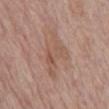Case summary:
* follow-up · total-body-photography surveillance lesion; no biopsy
* subject · male, aged approximately 70
* image-analysis metrics · an area of roughly 12 mm² and a shape-asymmetry score of about 0.45 (0 = symmetric); a border-irregularity rating of about 6/10, a within-lesion color-variation index near 3.5/10, and peripheral color asymmetry of about 1; a classifier nevus-likeness of about 0/100 and lesion-presence confidence of about 100/100
* body site · the front of the torso
* image source · ~15 mm tile from a whole-body skin photo
* lesion size · ~6.5 mm (longest diameter)